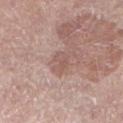{"image": {"source": "total-body photography crop", "field_of_view_mm": 15}, "patient": {"sex": "male", "age_approx": 55}, "site": "left lower leg", "lesion_size": {"long_diameter_mm_approx": 3.0}, "lighting": "white-light"}